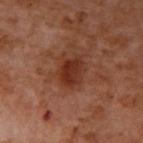No biopsy was performed on this lesion — it was imaged during a full skin examination and was not determined to be concerning.
Approximately 4 mm at its widest.
This is a cross-polarized tile.
A male patient aged around 55.
Automated tile analysis of the lesion measured an area of roughly 8.5 mm², an outline eccentricity of about 0.6 (0 = round, 1 = elongated), and two-axis asymmetry of about 0.2. The software also gave a normalized lesion–skin contrast near 8.5. The analysis additionally found a border-irregularity index near 2/10, internal color variation of about 4 on a 0–10 scale, and peripheral color asymmetry of about 1. It also reported a nevus-likeness score of about 70/100 and a lesion-detection confidence of about 100/100.
A region of skin cropped from a whole-body photographic capture, roughly 15 mm wide.
The lesion is located on the right upper arm.